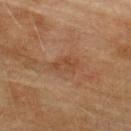Imaged during a routine full-body skin examination; the lesion was not biopsied and no histopathology is available. A male patient, aged around 75. The lesion is on the upper back. Automated image analysis of the tile measured a lesion area of about 5.5 mm² and a symmetry-axis asymmetry near 0.25. It also reported about 5 CIELAB-L* units darker than the surrounding skin and a lesion-to-skin contrast of about 5 (normalized; higher = more distinct). The software also gave a color-variation rating of about 2.5/10. Captured under cross-polarized illumination. Approximately 3 mm at its widest. A 15 mm crop from a total-body photograph taken for skin-cancer surveillance.